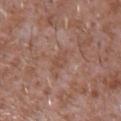Assessment:
The lesion was photographed on a routine skin check and not biopsied; there is no pathology result.
Clinical summary:
Longest diameter approximately 2.5 mm. From the chest. A roughly 15 mm field-of-view crop from a total-body skin photograph. A male subject, in their mid-40s.